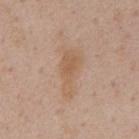Part of a total-body skin-imaging series; this lesion was reviewed on a skin check and was not flagged for biopsy. Measured at roughly 5 mm in maximum diameter. From the front of the torso. Imaged with white-light lighting. The subject is a male aged around 60. A 15 mm close-up tile from a total-body photography series done for melanoma screening. The lesion-visualizer software estimated a mean CIELAB color near L≈58 a*≈18 b*≈32, roughly 7 lightness units darker than nearby skin, and a normalized border contrast of about 6.